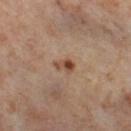{"biopsy_status": "not biopsied; imaged during a skin examination", "lighting": "cross-polarized", "site": "left thigh", "patient": {"sex": "female", "age_approx": 55}, "image": {"source": "total-body photography crop", "field_of_view_mm": 15}, "automated_metrics": {"area_mm2_approx": 3.5, "eccentricity": 0.85, "shape_asymmetry": 0.3, "cielab_L": 48, "cielab_a": 20, "cielab_b": 31, "vs_skin_darker_L": 11.0, "vs_skin_contrast_norm": 8.5, "nevus_likeness_0_100": 90}}